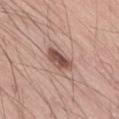Assessment: Recorded during total-body skin imaging; not selected for excision or biopsy. Acquisition and patient details: From the right thigh. A male subject roughly 55 years of age. A region of skin cropped from a whole-body photographic capture, roughly 15 mm wide.